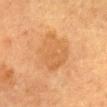Recorded during total-body skin imaging; not selected for excision or biopsy.
Measured at roughly 5 mm in maximum diameter.
A close-up tile cropped from a whole-body skin photograph, about 15 mm across.
The tile uses cross-polarized illumination.
A female patient, about 55 years old.
Located on the mid back.